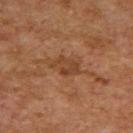– workup · no biopsy performed (imaged during a skin exam)
– patient · female, approximately 60 years of age
– site · the back
– lighting · cross-polarized
– automated lesion analysis · a footprint of about 5.5 mm², an eccentricity of roughly 0.8, and a symmetry-axis asymmetry near 0.35; a border-irregularity rating of about 4.5/10, internal color variation of about 2.5 on a 0–10 scale, and radial color variation of about 0.5
– acquisition · ~15 mm tile from a whole-body skin photo